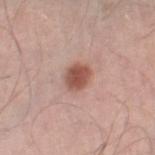location: the right lower leg | patient: male, in their mid-50s | image source: ~15 mm crop, total-body skin-cancer survey | image-analysis metrics: a lesion area of about 6.5 mm², an eccentricity of roughly 0.6, and two-axis asymmetry of about 0.2; a border-irregularity rating of about 1.5/10, a color-variation rating of about 3/10, and radial color variation of about 1; a classifier nevus-likeness of about 100/100 and lesion-presence confidence of about 100/100 | lesion size: about 3 mm.